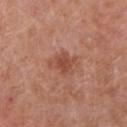The lesion was tiled from a total-body skin photograph and was not biopsied. The tile uses white-light illumination. The recorded lesion diameter is about 3.5 mm. On the right lower leg. An algorithmic analysis of the crop reported a footprint of about 6 mm², an outline eccentricity of about 0.65 (0 = round, 1 = elongated), and two-axis asymmetry of about 0.35. It also reported internal color variation of about 1.5 on a 0–10 scale and a peripheral color-asymmetry measure near 0.5. The subject is a male approximately 80 years of age. A 15 mm close-up extracted from a 3D total-body photography capture.